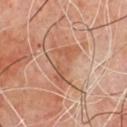{
  "biopsy_status": "not biopsied; imaged during a skin examination",
  "image": {
    "source": "total-body photography crop",
    "field_of_view_mm": 15
  },
  "site": "chest",
  "lighting": "cross-polarized",
  "lesion_size": {
    "long_diameter_mm_approx": 5.5
  },
  "patient": {
    "sex": "male",
    "age_approx": 65
  },
  "automated_metrics": {
    "vs_skin_darker_L": 8.0,
    "vs_skin_contrast_norm": 6.0,
    "color_variation_0_10": 4.0,
    "peripheral_color_asymmetry": 1.0,
    "nevus_likeness_0_100": 0,
    "lesion_detection_confidence_0_100": 90
  }
}